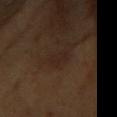The lesion was photographed on a routine skin check and not biopsied; there is no pathology result. On the chest. Captured under cross-polarized illumination. Automated image analysis of the tile measured an area of roughly 3 mm², an outline eccentricity of about 0.85 (0 = round, 1 = elongated), and a symmetry-axis asymmetry near 0.35. The software also gave a lesion color around L≈23 a*≈15 b*≈21 in CIELAB and a normalized lesion–skin contrast near 5. The analysis additionally found a border-irregularity index near 3.5/10, a color-variation rating of about 0/10, and a peripheral color-asymmetry measure near 0. Cropped from a whole-body photographic skin survey; the tile spans about 15 mm. A female patient aged 68–72.